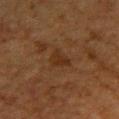| field | value |
|---|---|
| notes | catalogued during a skin exam; not biopsied |
| image source | ~15 mm crop, total-body skin-cancer survey |
| body site | the right upper arm |
| subject | female, about 55 years old |
| lighting | cross-polarized |